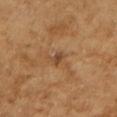Notes:
* notes: catalogued during a skin exam; not biopsied
* body site: the right upper arm
* subject: female, about 70 years old
* image source: ~15 mm tile from a whole-body skin photo
* tile lighting: cross-polarized